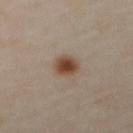This lesion was catalogued during total-body skin photography and was not selected for biopsy.
Located on the abdomen.
A 15 mm close-up extracted from a 3D total-body photography capture.
A male subject aged approximately 65.